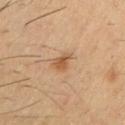No biopsy was performed on this lesion — it was imaged during a full skin examination and was not determined to be concerning. A close-up tile cropped from a whole-body skin photograph, about 15 mm across. The tile uses cross-polarized illumination. Located on the chest. A male subject, aged 58 to 62.